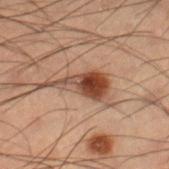A roughly 15 mm field-of-view crop from a total-body skin photograph.
From the right lower leg.
An algorithmic analysis of the crop reported an eccentricity of roughly 0.9 and two-axis asymmetry of about 0.6. The analysis additionally found an average lesion color of about L≈37 a*≈18 b*≈25 (CIELAB) and roughly 12 lightness units darker than nearby skin. The analysis additionally found a border-irregularity rating of about 7.5/10, a within-lesion color-variation index near 6.5/10, and a peripheral color-asymmetry measure near 2.
Imaged with cross-polarized lighting.
A male patient, in their mid- to late 50s.
About 6.5 mm across.